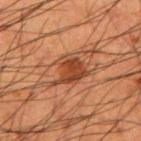Acquisition and patient details:
A region of skin cropped from a whole-body photographic capture, roughly 15 mm wide. The recorded lesion diameter is about 4.5 mm. This is a cross-polarized tile. A male subject, about 50 years old. The lesion is on the arm.